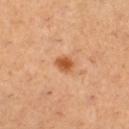Case summary:
– biopsy status · imaged on a skin check; not biopsied
– image source · 15 mm crop, total-body photography
– lesion diameter · ~2 mm (longest diameter)
– site · the right lower leg
– patient · female, aged 28 to 32